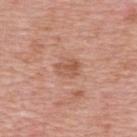| field | value |
|---|---|
| image-analysis metrics | a lesion area of about 5 mm², a shape eccentricity near 0.65, and a symmetry-axis asymmetry near 0.25; an average lesion color of about L≈56 a*≈23 b*≈31 (CIELAB), a lesion–skin lightness drop of about 9, and a normalized border contrast of about 6; border irregularity of about 2.5 on a 0–10 scale and a peripheral color-asymmetry measure near 1.5; an automated nevus-likeness rating near 0 out of 100 and a lesion-detection confidence of about 100/100 |
| lesion diameter | about 3 mm |
| anatomic site | the upper back |
| image source | ~15 mm crop, total-body skin-cancer survey |
| illumination | white-light illumination |
| subject | female, approximately 40 years of age |